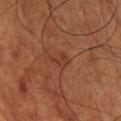follow-up: total-body-photography surveillance lesion; no biopsy
tile lighting: cross-polarized
lesion size: about 2.5 mm
patient: male, aged approximately 60
location: the chest
acquisition: 15 mm crop, total-body photography